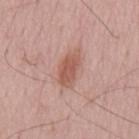The lesion was tiled from a total-body skin photograph and was not biopsied. Captured under white-light illumination. A close-up tile cropped from a whole-body skin photograph, about 15 mm across. A male subject, aged 53 to 57. The total-body-photography lesion software estimated a lesion area of about 7.5 mm², an outline eccentricity of about 0.85 (0 = round, 1 = elongated), and a symmetry-axis asymmetry near 0.25. The analysis additionally found an average lesion color of about L≈56 a*≈24 b*≈27 (CIELAB), roughly 10 lightness units darker than nearby skin, and a normalized lesion–skin contrast near 7.5. The software also gave a color-variation rating of about 2.5/10 and radial color variation of about 0.5. And it measured a nevus-likeness score of about 90/100 and lesion-presence confidence of about 100/100. The lesion's longest dimension is about 4.5 mm.